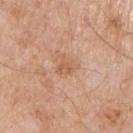The lesion was photographed on a routine skin check and not biopsied; there is no pathology result.
The patient is a male aged 63 to 67.
On the left upper arm.
Longest diameter approximately 2.5 mm.
Cropped from a whole-body photographic skin survey; the tile spans about 15 mm.
An algorithmic analysis of the crop reported a lesion area of about 4.5 mm² and an eccentricity of roughly 0.35.
Imaged with white-light lighting.